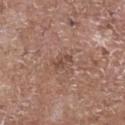The lesion was tiled from a total-body skin photograph and was not biopsied.
A lesion tile, about 15 mm wide, cut from a 3D total-body photograph.
A male subject, aged 68–72.
The tile uses white-light illumination.
The lesion is located on the chest.